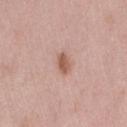Q: Is there a histopathology result?
A: catalogued during a skin exam; not biopsied